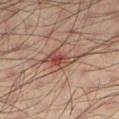Findings:
* notes — total-body-photography surveillance lesion; no biopsy
* lighting — cross-polarized
* subject — male, in their mid-30s
* automated metrics — border irregularity of about 4.5 on a 0–10 scale, a within-lesion color-variation index near 6/10, and peripheral color asymmetry of about 2
* body site — the left lower leg
* image source — ~15 mm tile from a whole-body skin photo